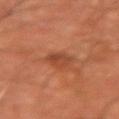<case>
<biopsy_status>not biopsied; imaged during a skin examination</biopsy_status>
<lighting>cross-polarized</lighting>
<site>arm</site>
<automated_metrics>
  <vs_skin_darker_L>7.0</vs_skin_darker_L>
  <vs_skin_contrast_norm>6.0</vs_skin_contrast_norm>
  <border_irregularity_0_10>2.0</border_irregularity_0_10>
  <color_variation_0_10>3.0</color_variation_0_10>
  <peripheral_color_asymmetry>1.0</peripheral_color_asymmetry>
  <nevus_likeness_0_100>0</nevus_likeness_0_100>
</automated_metrics>
<lesion_size>
  <long_diameter_mm_approx>2.5</long_diameter_mm_approx>
</lesion_size>
<image>
  <source>total-body photography crop</source>
  <field_of_view_mm>15</field_of_view_mm>
</image>
<patient>
  <sex>male</sex>
  <age_approx>65</age_approx>
</patient>
</case>